The tile uses cross-polarized illumination · located on the left upper arm · cropped from a total-body skin-imaging series; the visible field is about 15 mm · the lesion's longest dimension is about 5 mm · a female subject.
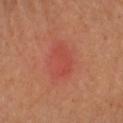Diagnosis: On excision, pathology confirmed a benign lesion: seborrheic keratosis.